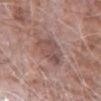Clinical impression: Imaged during a routine full-body skin examination; the lesion was not biopsied and no histopathology is available. Context: The lesion's longest dimension is about 4 mm. The lesion-visualizer software estimated a mean CIELAB color near L≈50 a*≈19 b*≈22 and a lesion–skin lightness drop of about 8. The analysis additionally found a border-irregularity rating of about 3/10, internal color variation of about 4 on a 0–10 scale, and a peripheral color-asymmetry measure near 1.5. It also reported a detector confidence of about 95 out of 100 that the crop contains a lesion. On the left forearm. A 15 mm close-up extracted from a 3D total-body photography capture. The subject is a male aged 73–77.